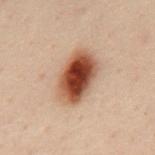<lesion>
<biopsy_status>not biopsied; imaged during a skin examination</biopsy_status>
<image>
  <source>total-body photography crop</source>
  <field_of_view_mm>15</field_of_view_mm>
</image>
<automated_metrics>
  <vs_skin_darker_L>17.0</vs_skin_darker_L>
  <vs_skin_contrast_norm>13.0</vs_skin_contrast_norm>
  <color_variation_0_10>9.0</color_variation_0_10>
  <peripheral_color_asymmetry>2.5</peripheral_color_asymmetry>
  <nevus_likeness_0_100>100</nevus_likeness_0_100>
</automated_metrics>
<lesion_size>
  <long_diameter_mm_approx>6.0</long_diameter_mm_approx>
</lesion_size>
<lighting>cross-polarized</lighting>
<site>mid back</site>
<patient>
  <sex>male</sex>
  <age_approx>50</age_approx>
</patient>
</lesion>